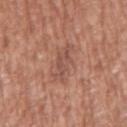Clinical impression:
The lesion was tiled from a total-body skin photograph and was not biopsied.
Acquisition and patient details:
From the right upper arm. This is a white-light tile. Approximately 5 mm at its widest. A male patient, roughly 75 years of age. A 15 mm close-up extracted from a 3D total-body photography capture.